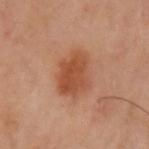Impression:
The lesion was tiled from a total-body skin photograph and was not biopsied.
Context:
Captured under cross-polarized illumination. A region of skin cropped from a whole-body photographic capture, roughly 15 mm wide. The lesion's longest dimension is about 5 mm. From the left upper arm.